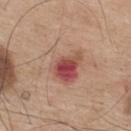Automated tile analysis of the lesion measured an eccentricity of roughly 0.75 and two-axis asymmetry of about 0.35. It also reported about 9 CIELAB-L* units darker than the surrounding skin and a lesion-to-skin contrast of about 6 (normalized; higher = more distinct). The recorded lesion diameter is about 7.5 mm. The lesion is located on the mid back. A lesion tile, about 15 mm wide, cut from a 3D total-body photograph. A male patient, approximately 75 years of age.